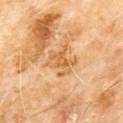Captured during whole-body skin photography for melanoma surveillance; the lesion was not biopsied. The total-body-photography lesion software estimated a border-irregularity index near 6/10, a color-variation rating of about 4.5/10, and peripheral color asymmetry of about 1.5. Located on the front of the torso. A male subject approximately 60 years of age. The tile uses cross-polarized illumination. A 15 mm close-up extracted from a 3D total-body photography capture. Approximately 3.5 mm at its widest.